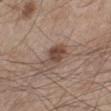Notes:
• biopsy status — no biopsy performed (imaged during a skin exam)
• size — about 3.5 mm
• acquisition — ~15 mm tile from a whole-body skin photo
• body site — the left lower leg
• lighting — white-light illumination
• subject — male, aged 43 to 47